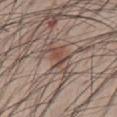Imaged during a routine full-body skin examination; the lesion was not biopsied and no histopathology is available.
A close-up tile cropped from a whole-body skin photograph, about 15 mm across.
Approximately 5 mm at its widest.
A male patient, about 40 years old.
From the abdomen.
The total-body-photography lesion software estimated a nevus-likeness score of about 90/100 and a lesion-detection confidence of about 85/100.
This is a white-light tile.